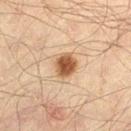{"biopsy_status": "not biopsied; imaged during a skin examination", "site": "left thigh", "automated_metrics": {"area_mm2_approx": 6.5, "eccentricity": 0.5, "shape_asymmetry": 0.2}, "image": {"source": "total-body photography crop", "field_of_view_mm": 15}, "lighting": "cross-polarized", "patient": {"sex": "male", "age_approx": 60}}